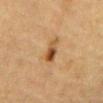| key | value |
|---|---|
| biopsy status | no biopsy performed (imaged during a skin exam) |
| site | the back |
| subject | male, aged around 85 or older |
| lighting | cross-polarized |
| acquisition | 15 mm crop, total-body photography |
| size | ~3 mm (longest diameter) |
| automated lesion analysis | an average lesion color of about L≈44 a*≈19 b*≈35 (CIELAB), roughly 11 lightness units darker than nearby skin, and a lesion-to-skin contrast of about 9 (normalized; higher = more distinct); a classifier nevus-likeness of about 90/100 and lesion-presence confidence of about 100/100 |